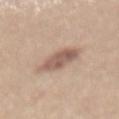  biopsy_status: not biopsied; imaged during a skin examination
  lighting: white-light
  site: back
  lesion_size:
    long_diameter_mm_approx: 4.5
  automated_metrics:
    cielab_L: 57
    cielab_a: 17
    cielab_b: 26
    vs_skin_darker_L: 12.0
    border_irregularity_0_10: 2.0
    color_variation_0_10: 3.5
    peripheral_color_asymmetry: 1.0
  patient:
    sex: female
    age_approx: 40
  image:
    source: total-body photography crop
    field_of_view_mm: 15